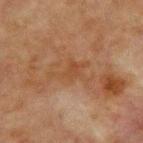Clinical impression:
The lesion was photographed on a routine skin check and not biopsied; there is no pathology result.
Background:
The total-body-photography lesion software estimated a footprint of about 6 mm², a shape eccentricity near 0.8, and a symmetry-axis asymmetry near 0.55. The analysis additionally found a mean CIELAB color near L≈38 a*≈18 b*≈29 and about 5 CIELAB-L* units darker than the surrounding skin. The analysis additionally found a nevus-likeness score of about 0/100 and a detector confidence of about 100 out of 100 that the crop contains a lesion. A male subject, aged 63–67. On the chest. A lesion tile, about 15 mm wide, cut from a 3D total-body photograph.